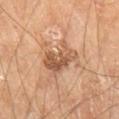| field | value |
|---|---|
| follow-up | catalogued during a skin exam; not biopsied |
| subject | male, aged 68–72 |
| tile lighting | cross-polarized illumination |
| location | the right thigh |
| acquisition | 15 mm crop, total-body photography |
| TBP lesion metrics | a lesion–skin lightness drop of about 11 and a normalized lesion–skin contrast near 7.5 |
| diameter | ≈5 mm |